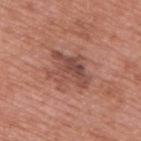This lesion was catalogued during total-body skin photography and was not selected for biopsy. A close-up tile cropped from a whole-body skin photograph, about 15 mm across. On the upper back. The lesion's longest dimension is about 5 mm. A male subject, roughly 70 years of age.